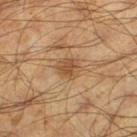Impression: Captured during whole-body skin photography for melanoma surveillance; the lesion was not biopsied. Image and clinical context: Captured under cross-polarized illumination. A lesion tile, about 15 mm wide, cut from a 3D total-body photograph. About 2.5 mm across. Located on the left thigh. A male subject, about 60 years old.